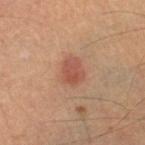Case summary:
- subject: male, approximately 40 years of age
- body site: the right lower leg
- image source: 15 mm crop, total-body photography
- automated lesion analysis: a lesion color around L≈46 a*≈22 b*≈27 in CIELAB and roughly 8 lightness units darker than nearby skin; an automated nevus-likeness rating near 75 out of 100 and a detector confidence of about 100 out of 100 that the crop contains a lesion
- size: about 3.5 mm